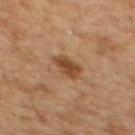Clinical impression: Recorded during total-body skin imaging; not selected for excision or biopsy.